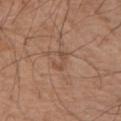Captured during whole-body skin photography for melanoma surveillance; the lesion was not biopsied. A male patient, roughly 60 years of age. The lesion is on the left upper arm. Cropped from a total-body skin-imaging series; the visible field is about 15 mm.